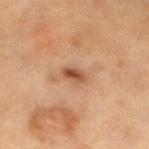Impression: This lesion was catalogued during total-body skin photography and was not selected for biopsy. Context: On the left lower leg. The patient is a female aged approximately 55. This image is a 15 mm lesion crop taken from a total-body photograph. The total-body-photography lesion software estimated border irregularity of about 2 on a 0–10 scale and internal color variation of about 3 on a 0–10 scale. Measured at roughly 3 mm in maximum diameter.